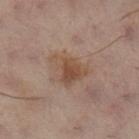follow-up: imaged on a skin check; not biopsied
anatomic site: the left leg
acquisition: ~15 mm crop, total-body skin-cancer survey
subject: female, aged around 55
tile lighting: cross-polarized